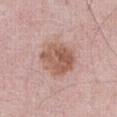This lesion was catalogued during total-body skin photography and was not selected for biopsy.
The tile uses white-light illumination.
The subject is a male about 60 years old.
The lesion is on the abdomen.
A close-up tile cropped from a whole-body skin photograph, about 15 mm across.